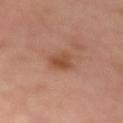Q: Was a biopsy performed?
A: no biopsy performed (imaged during a skin exam)
Q: How large is the lesion?
A: ≈3 mm
Q: Lesion location?
A: the left upper arm
Q: Who is the patient?
A: male, in their mid-60s
Q: What is the imaging modality?
A: 15 mm crop, total-body photography
Q: What did automated image analysis measure?
A: an area of roughly 4.5 mm², a shape eccentricity near 0.7, and two-axis asymmetry of about 0.25; a mean CIELAB color near L≈45 a*≈22 b*≈30, roughly 9 lightness units darker than nearby skin, and a normalized lesion–skin contrast near 7.5